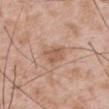<tbp_lesion>
<biopsy_status>not biopsied; imaged during a skin examination</biopsy_status>
<site>mid back</site>
<patient>
  <sex>male</sex>
  <age_approx>50</age_approx>
</patient>
<lighting>white-light</lighting>
<automated_metrics>
  <cielab_L>57</cielab_L>
  <cielab_a>20</cielab_a>
  <cielab_b>30</cielab_b>
  <vs_skin_darker_L>9.0</vs_skin_darker_L>
  <color_variation_0_10>2.0</color_variation_0_10>
  <peripheral_color_asymmetry>1.0</peripheral_color_asymmetry>
  <nevus_likeness_0_100>10</nevus_likeness_0_100>
  <lesion_detection_confidence_0_100>100</lesion_detection_confidence_0_100>
</automated_metrics>
<image>
  <source>total-body photography crop</source>
  <field_of_view_mm>15</field_of_view_mm>
</image>
<lesion_size>
  <long_diameter_mm_approx>3.5</long_diameter_mm_approx>
</lesion_size>
</tbp_lesion>